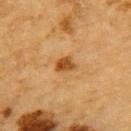- workup — catalogued during a skin exam; not biopsied
- acquisition — ~15 mm crop, total-body skin-cancer survey
- diameter — ~2.5 mm (longest diameter)
- patient — male, in their mid- to late 80s or older
- site — the upper back
- automated lesion analysis — an outline eccentricity of about 0.7 (0 = round, 1 = elongated) and two-axis asymmetry of about 0.3; a classifier nevus-likeness of about 90/100 and a lesion-detection confidence of about 100/100